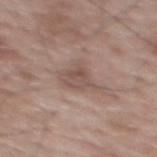| key | value |
|---|---|
| workup | total-body-photography surveillance lesion; no biopsy |
| lesion diameter | ≈4.5 mm |
| illumination | white-light |
| subject | male, aged approximately 70 |
| acquisition | ~15 mm crop, total-body skin-cancer survey |
| anatomic site | the back |